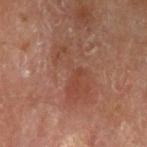{
  "biopsy_status": "not biopsied; imaged during a skin examination",
  "site": "left upper arm",
  "patient": {
    "sex": "male",
    "age_approx": "85+"
  },
  "lighting": "cross-polarized",
  "lesion_size": {
    "long_diameter_mm_approx": 6.5
  },
  "image": {
    "source": "total-body photography crop",
    "field_of_view_mm": 15
  }
}